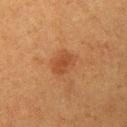The lesion was photographed on a routine skin check and not biopsied; there is no pathology result.
About 3.5 mm across.
This is a cross-polarized tile.
The total-body-photography lesion software estimated a lesion area of about 7 mm², an eccentricity of roughly 0.7, and a shape-asymmetry score of about 0.15 (0 = symmetric). It also reported a border-irregularity index near 2/10, a within-lesion color-variation index near 2/10, and peripheral color asymmetry of about 1.
A 15 mm close-up extracted from a 3D total-body photography capture.
The subject is a female about 40 years old.
Located on the left upper arm.